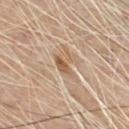Impression:
This lesion was catalogued during total-body skin photography and was not selected for biopsy.
Background:
Cropped from a whole-body photographic skin survey; the tile spans about 15 mm. Captured under cross-polarized illumination. Approximately 3 mm at its widest. Located on the chest. Automated tile analysis of the lesion measured a footprint of about 4.5 mm², an eccentricity of roughly 0.75, and a shape-asymmetry score of about 0.4 (0 = symmetric). The analysis additionally found a border-irregularity index near 4.5/10, internal color variation of about 4.5 on a 0–10 scale, and a peripheral color-asymmetry measure near 2. A male patient, roughly 65 years of age.